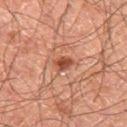Image and clinical context: On the right thigh. A roughly 15 mm field-of-view crop from a total-body skin photograph. The tile uses cross-polarized illumination. Automated tile analysis of the lesion measured a lesion color around L≈44 a*≈26 b*≈30 in CIELAB and about 12 CIELAB-L* units darker than the surrounding skin. A male subject, about 60 years old.